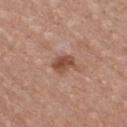Notes:
– follow-up · no biopsy performed (imaged during a skin exam)
– patient · female, aged 33 to 37
– imaging modality · ~15 mm tile from a whole-body skin photo
– TBP lesion metrics · border irregularity of about 2.5 on a 0–10 scale and peripheral color asymmetry of about 1; a classifier nevus-likeness of about 55/100 and lesion-presence confidence of about 100/100
– anatomic site · the chest
– diameter · about 3 mm